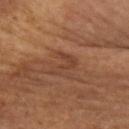Context:
Captured under cross-polarized illumination. A region of skin cropped from a whole-body photographic capture, roughly 15 mm wide. The lesion is on the right forearm. Automated image analysis of the tile measured a lesion color around L≈38 a*≈20 b*≈28 in CIELAB, about 6 CIELAB-L* units darker than the surrounding skin, and a normalized lesion–skin contrast near 5. And it measured a within-lesion color-variation index near 1/10. A female patient, roughly 60 years of age.